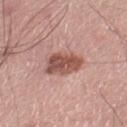Part of a total-body skin-imaging series; this lesion was reviewed on a skin check and was not flagged for biopsy. The patient is a male about 70 years old. On the left thigh. This image is a 15 mm lesion crop taken from a total-body photograph. Imaged with white-light lighting. Approximately 4 mm at its widest.